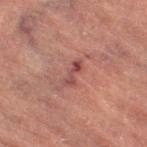Clinical impression: The lesion was photographed on a routine skin check and not biopsied; there is no pathology result. Acquisition and patient details: The lesion is on the right thigh. Automated tile analysis of the lesion measured a footprint of about 2.5 mm² and a shape eccentricity near 0.95. It also reported a border-irregularity index near 6/10 and internal color variation of about 0 on a 0–10 scale. The analysis additionally found a lesion-detection confidence of about 95/100. Captured under cross-polarized illumination. Cropped from a total-body skin-imaging series; the visible field is about 15 mm. A female patient, in their 70s.